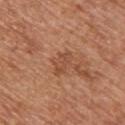This lesion was catalogued during total-body skin photography and was not selected for biopsy.
Approximately 3 mm at its widest.
A male patient, aged 63 to 67.
The lesion is located on the upper back.
Automated image analysis of the tile measured an area of roughly 4.5 mm², a shape eccentricity near 0.75, and a symmetry-axis asymmetry near 0.3. And it measured a mean CIELAB color near L≈49 a*≈24 b*≈33 and a normalized lesion–skin contrast near 5.5. The software also gave a border-irregularity index near 3.5/10 and a within-lesion color-variation index near 1.5/10. The analysis additionally found a nevus-likeness score of about 0/100.
This image is a 15 mm lesion crop taken from a total-body photograph.